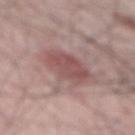workup = imaged on a skin check; not biopsied.